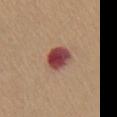Q: Is there a histopathology result?
A: imaged on a skin check; not biopsied
Q: How was this image acquired?
A: 15 mm crop, total-body photography
Q: Where on the body is the lesion?
A: the chest
Q: What are the patient's age and sex?
A: female, aged 58–62